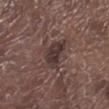Imaged during a routine full-body skin examination; the lesion was not biopsied and no histopathology is available. A male patient, aged 68 to 72. From the leg. A close-up tile cropped from a whole-body skin photograph, about 15 mm across.